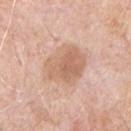biopsy status=imaged on a skin check; not biopsied | anatomic site=the chest | automated metrics=a lesion color around L≈64 a*≈20 b*≈31 in CIELAB, roughly 9 lightness units darker than nearby skin, and a normalized border contrast of about 6.5; a nevus-likeness score of about 0/100 and a lesion-detection confidence of about 100/100 | image source=15 mm crop, total-body photography | patient=male, about 70 years old | lesion size=~5.5 mm (longest diameter).